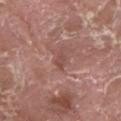| field | value |
|---|---|
| follow-up | total-body-photography surveillance lesion; no biopsy |
| tile lighting | white-light illumination |
| image-analysis metrics | a lesion color around L≈48 a*≈22 b*≈25 in CIELAB and about 7 CIELAB-L* units darker than the surrounding skin; border irregularity of about 3 on a 0–10 scale, a color-variation rating of about 0.5/10, and radial color variation of about 0 |
| lesion diameter | about 2.5 mm |
| image | ~15 mm tile from a whole-body skin photo |
| subject | male, roughly 40 years of age |
| location | the right thigh |